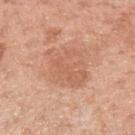Part of a total-body skin-imaging series; this lesion was reviewed on a skin check and was not flagged for biopsy.
Located on the left forearm.
The lesion-visualizer software estimated a lesion color around L≈61 a*≈23 b*≈32 in CIELAB, a lesion–skin lightness drop of about 7, and a normalized lesion–skin contrast near 5. It also reported a border-irregularity rating of about 3/10.
Imaged with white-light lighting.
Longest diameter approximately 5 mm.
A lesion tile, about 15 mm wide, cut from a 3D total-body photograph.
A female patient aged 48–52.